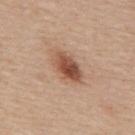follow-up = catalogued during a skin exam; not biopsied
imaging modality = 15 mm crop, total-body photography
anatomic site = the mid back
subject = male, approximately 65 years of age